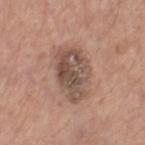Findings:
• follow-up — imaged on a skin check; not biopsied
• anatomic site — the leg
• subject — male, aged around 65
• tile lighting — white-light
• imaging modality — total-body-photography crop, ~15 mm field of view